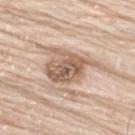Q: Was a biopsy performed?
A: imaged on a skin check; not biopsied
Q: What is the anatomic site?
A: the upper back
Q: How was this image acquired?
A: total-body-photography crop, ~15 mm field of view
Q: Automated lesion metrics?
A: a lesion area of about 17 mm², an outline eccentricity of about 0.7 (0 = round, 1 = elongated), and a shape-asymmetry score of about 0.4 (0 = symmetric); an average lesion color of about L≈61 a*≈16 b*≈29 (CIELAB), a lesion–skin lightness drop of about 13, and a lesion-to-skin contrast of about 8.5 (normalized; higher = more distinct)
Q: Lesion size?
A: ~6.5 mm (longest diameter)
Q: What are the patient's age and sex?
A: male, aged 73–77